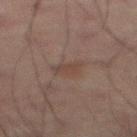Notes:
– follow-up — imaged on a skin check; not biopsied
– body site — the mid back
– patient — male, aged approximately 65
– imaging modality — ~15 mm tile from a whole-body skin photo
– size — ≈2.5 mm
– automated metrics — a mean CIELAB color near L≈33 a*≈14 b*≈19, about 5 CIELAB-L* units darker than the surrounding skin, and a normalized border contrast of about 5; a classifier nevus-likeness of about 10/100 and lesion-presence confidence of about 100/100
– lighting — cross-polarized illumination